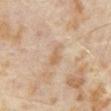Findings:
• notes: no biopsy performed (imaged during a skin exam)
• lighting: cross-polarized
• size: ~2.5 mm (longest diameter)
• patient: male, about 65 years old
• acquisition: ~15 mm tile from a whole-body skin photo